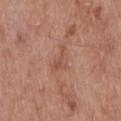<record>
<biopsy_status>not biopsied; imaged during a skin examination</biopsy_status>
</record>